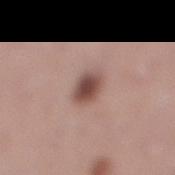The subject is a female aged 38–42.
Measured at roughly 3 mm in maximum diameter.
The lesion is on the right lower leg.
Automated tile analysis of the lesion measured an area of roughly 6 mm², an eccentricity of roughly 0.5, and a symmetry-axis asymmetry near 0.15.
A 15 mm crop from a total-body photograph taken for skin-cancer surveillance.
The tile uses white-light illumination.